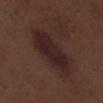This lesion was catalogued during total-body skin photography and was not selected for biopsy. The recorded lesion diameter is about 8.5 mm. A male subject, aged 68 to 72. Automated image analysis of the tile measured a footprint of about 22 mm², a shape eccentricity near 0.9, and two-axis asymmetry of about 0.2. This image is a 15 mm lesion crop taken from a total-body photograph. From the right lower leg.